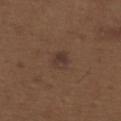Captured during whole-body skin photography for melanoma surveillance; the lesion was not biopsied.